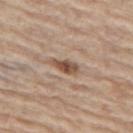| field | value |
|---|---|
| notes | no biopsy performed (imaged during a skin exam) |
| location | the left thigh |
| automated lesion analysis | an average lesion color of about L≈51 a*≈17 b*≈28 (CIELAB) and roughly 13 lightness units darker than nearby skin; a border-irregularity rating of about 3/10, a within-lesion color-variation index near 3.5/10, and radial color variation of about 1 |
| tile lighting | white-light |
| patient | male, roughly 70 years of age |
| image | ~15 mm crop, total-body skin-cancer survey |